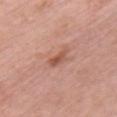{
  "biopsy_status": "not biopsied; imaged during a skin examination",
  "patient": {
    "sex": "female",
    "age_approx": 65
  },
  "lighting": "white-light",
  "image": {
    "source": "total-body photography crop",
    "field_of_view_mm": 15
  },
  "site": "chest",
  "lesion_size": {
    "long_diameter_mm_approx": 3.0
  }
}